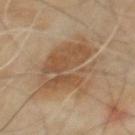Clinical impression:
No biopsy was performed on this lesion — it was imaged during a full skin examination and was not determined to be concerning.
Acquisition and patient details:
A 15 mm crop from a total-body photograph taken for skin-cancer surveillance. Captured under cross-polarized illumination. The lesion's longest dimension is about 8 mm. The subject is a male aged approximately 60. The lesion is on the mid back.